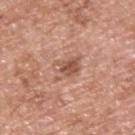  biopsy_status: not biopsied; imaged during a skin examination
  image:
    source: total-body photography crop
    field_of_view_mm: 15
  site: upper back
  patient:
    sex: male
    age_approx: 60